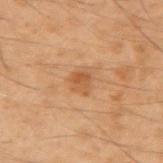No biopsy was performed on this lesion — it was imaged during a full skin examination and was not determined to be concerning.
This is a cross-polarized tile.
From the right upper arm.
The patient is a male approximately 50 years of age.
Cropped from a total-body skin-imaging series; the visible field is about 15 mm.
The recorded lesion diameter is about 2.5 mm.